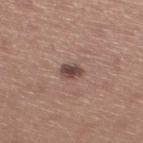Notes:
- imaging modality · ~15 mm crop, total-body skin-cancer survey
- site · the left lower leg
- illumination · white-light
- patient · male, roughly 60 years of age
- lesion diameter · about 2.5 mm
- TBP lesion metrics · border irregularity of about 2.5 on a 0–10 scale and peripheral color asymmetry of about 1